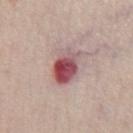notes: no biopsy performed (imaged during a skin exam)
location: the chest
imaging modality: 15 mm crop, total-body photography
diameter: ≈4.5 mm
lighting: white-light illumination
TBP lesion metrics: an eccentricity of roughly 0.8 and a shape-asymmetry score of about 0.2 (0 = symmetric)
subject: male, roughly 60 years of age